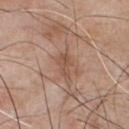workup = imaged on a skin check; not biopsied | body site = the chest | tile lighting = white-light | image = ~15 mm tile from a whole-body skin photo | patient = male, aged approximately 55 | lesion diameter = ≈3 mm.